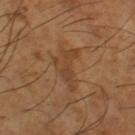Clinical impression: This lesion was catalogued during total-body skin photography and was not selected for biopsy. Image and clinical context: The lesion is located on the left thigh. The total-body-photography lesion software estimated an area of roughly 8 mm² and a symmetry-axis asymmetry near 0.6. The analysis additionally found roughly 6 lightness units darker than nearby skin. The tile uses cross-polarized illumination. A male patient aged 63 to 67. A close-up tile cropped from a whole-body skin photograph, about 15 mm across. The lesion's longest dimension is about 5 mm.